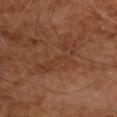biopsy_status: not biopsied; imaged during a skin examination
automated_metrics:
  area_mm2_approx: 8.5
  eccentricity: 0.95
  shape_asymmetry: 0.5
  cielab_L: 36
  cielab_a: 21
  cielab_b: 29
  vs_skin_darker_L: 5.0
  nevus_likeness_0_100: 0
image:
  source: total-body photography crop
  field_of_view_mm: 15
site: upper back
lesion_size:
  long_diameter_mm_approx: 7.0
lighting: cross-polarized
patient:
  sex: male
  age_approx: 70